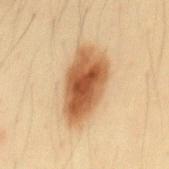Assessment:
The lesion was photographed on a routine skin check and not biopsied; there is no pathology result.
Image and clinical context:
Automated tile analysis of the lesion measured a footprint of about 24 mm², an eccentricity of roughly 0.85, and a shape-asymmetry score of about 0.2 (0 = symmetric). The analysis additionally found a border-irregularity index near 2.5/10, a color-variation rating of about 7.5/10, and a peripheral color-asymmetry measure near 2. The software also gave a nevus-likeness score of about 100/100. The patient is a male in their mid- to late 30s. The lesion is located on the mid back. Measured at roughly 8 mm in maximum diameter. Imaged with cross-polarized lighting. A lesion tile, about 15 mm wide, cut from a 3D total-body photograph.